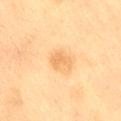Recorded during total-body skin imaging; not selected for excision or biopsy.
A male subject, in their mid- to late 50s.
The lesion-visualizer software estimated a classifier nevus-likeness of about 25/100 and a lesion-detection confidence of about 100/100.
Imaged with cross-polarized lighting.
A close-up tile cropped from a whole-body skin photograph, about 15 mm across.
On the lower back.